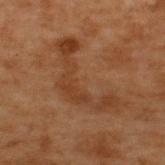| field | value |
|---|---|
| follow-up | catalogued during a skin exam; not biopsied |
| lesion diameter | ~8.5 mm (longest diameter) |
| image source | ~15 mm tile from a whole-body skin photo |
| patient | male, aged around 70 |
| site | the back |
| illumination | cross-polarized |